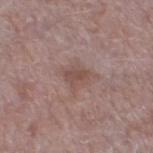{"biopsy_status": "not biopsied; imaged during a skin examination", "image": {"source": "total-body photography crop", "field_of_view_mm": 15}, "site": "left thigh", "lighting": "white-light", "lesion_size": {"long_diameter_mm_approx": 3.0}, "patient": {"sex": "male", "age_approx": 70}}